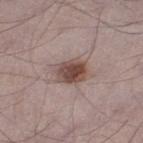The lesion was photographed on a routine skin check and not biopsied; there is no pathology result. A close-up tile cropped from a whole-body skin photograph, about 15 mm across. A male patient aged approximately 70. Imaged with white-light lighting. Measured at roughly 3.5 mm in maximum diameter. Automated image analysis of the tile measured a symmetry-axis asymmetry near 0.2. And it measured border irregularity of about 2 on a 0–10 scale, internal color variation of about 5 on a 0–10 scale, and radial color variation of about 1.5. The lesion is located on the left thigh.